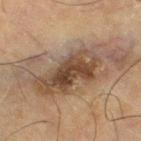Clinical impression: Imaged during a routine full-body skin examination; the lesion was not biopsied and no histopathology is available. Acquisition and patient details: A region of skin cropped from a whole-body photographic capture, roughly 15 mm wide. About 14.5 mm across. Imaged with cross-polarized lighting. The subject is a male about 65 years old. Automated tile analysis of the lesion measured border irregularity of about 5 on a 0–10 scale and radial color variation of about 2. And it measured a classifier nevus-likeness of about 15/100 and lesion-presence confidence of about 90/100. Located on the right thigh.